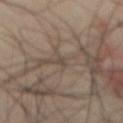Q: Was a biopsy performed?
A: no biopsy performed (imaged during a skin exam)
Q: Lesion size?
A: ~1.5 mm (longest diameter)
Q: How was the tile lit?
A: cross-polarized illumination
Q: What is the imaging modality?
A: total-body-photography crop, ~15 mm field of view
Q: Who is the patient?
A: male, aged 38 to 42
Q: Lesion location?
A: the mid back